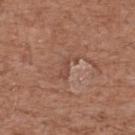Findings:
- workup: total-body-photography surveillance lesion; no biopsy
- site: the upper back
- image: total-body-photography crop, ~15 mm field of view
- patient: male, about 75 years old